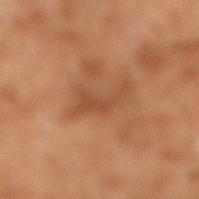Imaged during a routine full-body skin examination; the lesion was not biopsied and no histopathology is available. Imaged with cross-polarized lighting. The lesion's longest dimension is about 5 mm. A 15 mm close-up tile from a total-body photography series done for melanoma screening. A male subject, aged 63 to 67.